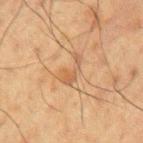Clinical summary: A lesion tile, about 15 mm wide, cut from a 3D total-body photograph. The subject is a male in their 60s. On the left upper arm.